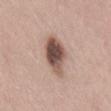patient = female, in their mid- to late 60s; TBP lesion metrics = an eccentricity of roughly 0.7; lighting = white-light; anatomic site = the abdomen; size = ~4.5 mm (longest diameter); image = ~15 mm crop, total-body skin-cancer survey.